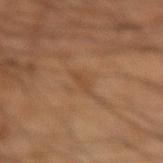biopsy status: catalogued during a skin exam; not biopsied | patient: male, in their mid- to late 40s | body site: the right forearm | lesion diameter: ≈2.5 mm | tile lighting: cross-polarized illumination | acquisition: 15 mm crop, total-body photography.